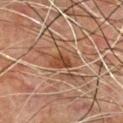Impression: Part of a total-body skin-imaging series; this lesion was reviewed on a skin check and was not flagged for biopsy. Acquisition and patient details: A 15 mm close-up extracted from a 3D total-body photography capture. Imaged with cross-polarized lighting. On the front of the torso. The total-body-photography lesion software estimated an average lesion color of about L≈36 a*≈20 b*≈28 (CIELAB), a lesion–skin lightness drop of about 8, and a lesion-to-skin contrast of about 8.5 (normalized; higher = more distinct). The analysis additionally found a border-irregularity rating of about 3.5/10, internal color variation of about 3.5 on a 0–10 scale, and a peripheral color-asymmetry measure near 1. It also reported lesion-presence confidence of about 95/100. The patient is a male in their mid-60s.